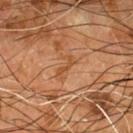{
  "biopsy_status": "not biopsied; imaged during a skin examination",
  "image": {
    "source": "total-body photography crop",
    "field_of_view_mm": 15
  },
  "patient": {
    "sex": "male",
    "age_approx": 55
  },
  "lesion_size": {
    "long_diameter_mm_approx": 3.0
  },
  "site": "chest",
  "automated_metrics": {
    "cielab_L": 46,
    "cielab_a": 23,
    "cielab_b": 36,
    "vs_skin_darker_L": 7.0,
    "vs_skin_contrast_norm": 6.0
  }
}